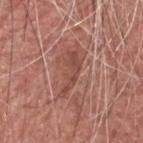{"biopsy_status": "not biopsied; imaged during a skin examination", "patient": {"sex": "male", "age_approx": 75}, "lesion_size": {"long_diameter_mm_approx": 5.0}, "site": "head or neck", "image": {"source": "total-body photography crop", "field_of_view_mm": 15}, "automated_metrics": {"border_irregularity_0_10": 7.5, "color_variation_0_10": 1.0, "peripheral_color_asymmetry": 0.5}, "lighting": "white-light"}